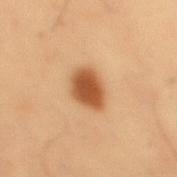Impression: Captured during whole-body skin photography for melanoma surveillance; the lesion was not biopsied. Acquisition and patient details: The total-body-photography lesion software estimated a lesion area of about 9 mm², an outline eccentricity of about 0.65 (0 = round, 1 = elongated), and a shape-asymmetry score of about 0.2 (0 = symmetric). It also reported border irregularity of about 1.5 on a 0–10 scale, a color-variation rating of about 3/10, and peripheral color asymmetry of about 1. And it measured a nevus-likeness score of about 100/100 and lesion-presence confidence of about 100/100. A male subject in their mid-50s. About 4 mm across. A roughly 15 mm field-of-view crop from a total-body skin photograph. Located on the mid back.